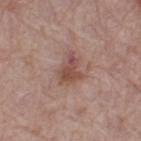{
  "biopsy_status": "not biopsied; imaged during a skin examination",
  "patient": {
    "sex": "female",
    "age_approx": 65
  },
  "site": "leg",
  "lesion_size": {
    "long_diameter_mm_approx": 4.0
  },
  "automated_metrics": {
    "area_mm2_approx": 6.5,
    "eccentricity": 0.75,
    "shape_asymmetry": 0.35,
    "cielab_L": 49,
    "cielab_a": 21,
    "cielab_b": 25,
    "vs_skin_darker_L": 10.0,
    "vs_skin_contrast_norm": 7.5
  },
  "image": {
    "source": "total-body photography crop",
    "field_of_view_mm": 15
  },
  "lighting": "white-light"
}